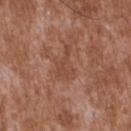The lesion was photographed on a routine skin check and not biopsied; there is no pathology result.
Captured under white-light illumination.
About 4 mm across.
The patient is a male aged around 45.
A 15 mm close-up tile from a total-body photography series done for melanoma screening.
Located on the upper back.
Automated tile analysis of the lesion measured an eccentricity of roughly 0.85 and a symmetry-axis asymmetry near 0.65. It also reported a lesion color around L≈46 a*≈23 b*≈30 in CIELAB, roughly 6 lightness units darker than nearby skin, and a lesion-to-skin contrast of about 5 (normalized; higher = more distinct). The software also gave a nevus-likeness score of about 0/100.